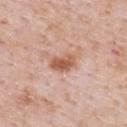Q: Was this lesion biopsied?
A: no biopsy performed (imaged during a skin exam)
Q: What are the patient's age and sex?
A: male, aged around 50
Q: Where on the body is the lesion?
A: the back
Q: Illumination type?
A: white-light illumination
Q: Lesion size?
A: ~3 mm (longest diameter)
Q: Automated lesion metrics?
A: a lesion area of about 6 mm², an eccentricity of roughly 0.7, and two-axis asymmetry of about 0.2
Q: What kind of image is this?
A: 15 mm crop, total-body photography